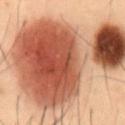Assessment: The lesion was photographed on a routine skin check and not biopsied; there is no pathology result. Image and clinical context: On the abdomen. The subject is a male aged around 55. Cropped from a whole-body photographic skin survey; the tile spans about 15 mm.